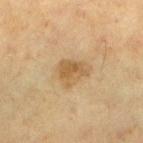  biopsy_status: not biopsied; imaged during a skin examination
  site: left lower leg
  image:
    source: total-body photography crop
    field_of_view_mm: 15
  patient:
    sex: female
    age_approx: 50
  lesion_size:
    long_diameter_mm_approx: 3.5
  lighting: cross-polarized